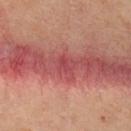Notes:
* workup · no biopsy performed (imaged during a skin exam)
* anatomic site · the back
* illumination · cross-polarized illumination
* imaging modality · total-body-photography crop, ~15 mm field of view
* subject · female, aged 43–47
* lesion diameter · ≈2.5 mm